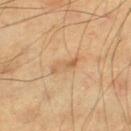Recorded during total-body skin imaging; not selected for excision or biopsy. A male subject aged approximately 70. A 15 mm close-up extracted from a 3D total-body photography capture. Measured at roughly 3.5 mm in maximum diameter. The lesion is on the left thigh.